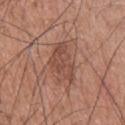Clinical impression: No biopsy was performed on this lesion — it was imaged during a full skin examination and was not determined to be concerning. Context: On the head or neck. The patient is a male aged 53 to 57. A region of skin cropped from a whole-body photographic capture, roughly 15 mm wide.